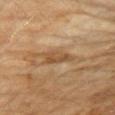No biopsy was performed on this lesion — it was imaged during a full skin examination and was not determined to be concerning.
Located on the right upper arm.
A female subject, aged 58 to 62.
A 15 mm crop from a total-body photograph taken for skin-cancer surveillance.